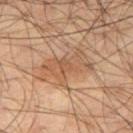Imaged during a routine full-body skin examination; the lesion was not biopsied and no histopathology is available.
On the right thigh.
Automated image analysis of the tile measured a within-lesion color-variation index near 3.5/10 and radial color variation of about 1. And it measured a nevus-likeness score of about 15/100 and a lesion-detection confidence of about 95/100.
Captured under cross-polarized illumination.
The recorded lesion diameter is about 5.5 mm.
The patient is a male aged around 55.
A lesion tile, about 15 mm wide, cut from a 3D total-body photograph.